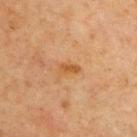Findings:
* biopsy status — no biopsy performed (imaged during a skin exam)